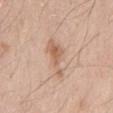Notes:
- notes — no biopsy performed (imaged during a skin exam)
- body site — the mid back
- tile lighting — white-light
- subject — male, in their 60s
- imaging modality — ~15 mm tile from a whole-body skin photo
- lesion diameter — about 5 mm